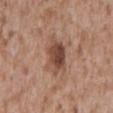Clinical impression: Imaged during a routine full-body skin examination; the lesion was not biopsied and no histopathology is available. Acquisition and patient details: This is a white-light tile. The subject is a male in their mid- to late 40s. The lesion's longest dimension is about 4.5 mm. Located on the mid back. This image is a 15 mm lesion crop taken from a total-body photograph.